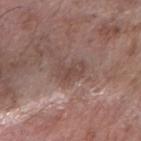  biopsy_status: not biopsied; imaged during a skin examination
  lesion_size:
    long_diameter_mm_approx: 3.5
  image:
    source: total-body photography crop
    field_of_view_mm: 15
  patient:
    sex: male
    age_approx: 60
  site: right forearm
  automated_metrics:
    area_mm2_approx: 6.5
    eccentricity: 0.7
    shape_asymmetry: 0.4
    lesion_detection_confidence_0_100: 100